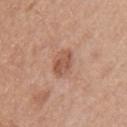Clinical impression:
Captured during whole-body skin photography for melanoma surveillance; the lesion was not biopsied.